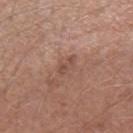Clinical impression:
Part of a total-body skin-imaging series; this lesion was reviewed on a skin check and was not flagged for biopsy.
Context:
On the left forearm. A 15 mm close-up tile from a total-body photography series done for melanoma screening. The lesion-visualizer software estimated an area of roughly 3 mm², an outline eccentricity of about 0.9 (0 = round, 1 = elongated), and a symmetry-axis asymmetry near 0.4. And it measured a detector confidence of about 100 out of 100 that the crop contains a lesion. Captured under white-light illumination. Measured at roughly 3 mm in maximum diameter. A female patient, approximately 60 years of age.